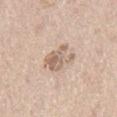follow-up = catalogued during a skin exam; not biopsied | body site = the right thigh | image = total-body-photography crop, ~15 mm field of view | patient = male, approximately 65 years of age.